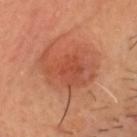  biopsy_status: not biopsied; imaged during a skin examination
  image:
    source: total-body photography crop
    field_of_view_mm: 15
  patient:
    sex: male
    age_approx: 45
  site: head or neck